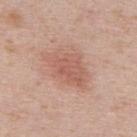Findings:
– notes · total-body-photography surveillance lesion; no biopsy
– automated lesion analysis · a classifier nevus-likeness of about 30/100 and a lesion-detection confidence of about 100/100
– lesion size · ~6 mm (longest diameter)
– patient · male, about 40 years old
– location · the upper back
– lighting · white-light
– image source · total-body-photography crop, ~15 mm field of view